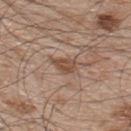Part of a total-body skin-imaging series; this lesion was reviewed on a skin check and was not flagged for biopsy.
The lesion is located on the back.
The subject is a male in their 50s.
Imaged with white-light lighting.
A 15 mm close-up tile from a total-body photography series done for melanoma screening.